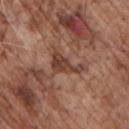No biopsy was performed on this lesion — it was imaged during a full skin examination and was not determined to be concerning. The subject is a male aged 68–72. The lesion is located on the chest. This image is a 15 mm lesion crop taken from a total-body photograph.